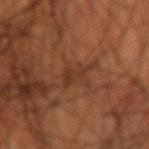* workup — total-body-photography surveillance lesion; no biopsy
* acquisition — ~15 mm crop, total-body skin-cancer survey
* body site — the right forearm
* TBP lesion metrics — a shape eccentricity near 0.9 and a symmetry-axis asymmetry near 0.65; a nevus-likeness score of about 0/100 and a detector confidence of about 60 out of 100 that the crop contains a lesion
* lesion diameter — ~4 mm (longest diameter)
* subject — male, roughly 55 years of age